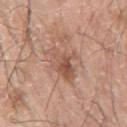image source=15 mm crop, total-body photography; body site=the left arm; patient=male, aged 68 to 72.